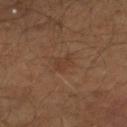Impression: The lesion was photographed on a routine skin check and not biopsied; there is no pathology result. Acquisition and patient details: Imaged with cross-polarized lighting. A male patient aged around 40. The lesion-visualizer software estimated a lesion color around L≈38 a*≈19 b*≈29 in CIELAB and roughly 5 lightness units darker than nearby skin. The software also gave peripheral color asymmetry of about 0.5. The lesion is on the right lower leg. About 2.5 mm across. Cropped from a total-body skin-imaging series; the visible field is about 15 mm.